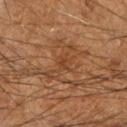follow-up: catalogued during a skin exam; not biopsied
automated lesion analysis: an outline eccentricity of about 0.8 (0 = round, 1 = elongated) and a shape-asymmetry score of about 0.4 (0 = symmetric); an average lesion color of about L≈37 a*≈22 b*≈32 (CIELAB), about 5 CIELAB-L* units darker than the surrounding skin, and a lesion-to-skin contrast of about 5 (normalized; higher = more distinct); border irregularity of about 3.5 on a 0–10 scale, a color-variation rating of about 0/10, and a peripheral color-asymmetry measure near 0
site: the right forearm
imaging modality: ~15 mm crop, total-body skin-cancer survey
patient: male, roughly 60 years of age
illumination: cross-polarized illumination